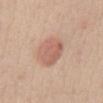No biopsy was performed on this lesion — it was imaged during a full skin examination and was not determined to be concerning. A region of skin cropped from a whole-body photographic capture, roughly 15 mm wide. The subject is a female about 50 years old. From the abdomen.